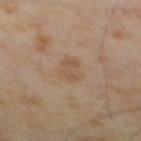No biopsy was performed on this lesion — it was imaged during a full skin examination and was not determined to be concerning.
A 15 mm crop from a total-body photograph taken for skin-cancer surveillance.
Measured at roughly 3.5 mm in maximum diameter.
The tile uses cross-polarized illumination.
The patient is a male aged 63 to 67.
Automated tile analysis of the lesion measured an eccentricity of roughly 0.7 and a symmetry-axis asymmetry near 0.3. And it measured a color-variation rating of about 2/10 and peripheral color asymmetry of about 0.5. The analysis additionally found a lesion-detection confidence of about 100/100.